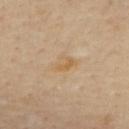Q: Was this lesion biopsied?
A: no biopsy performed (imaged during a skin exam)
Q: Lesion location?
A: the upper back
Q: What are the patient's age and sex?
A: female, aged 58–62
Q: What is the imaging modality?
A: ~15 mm crop, total-body skin-cancer survey
Q: How large is the lesion?
A: ~3 mm (longest diameter)